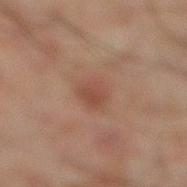follow-up: no biopsy performed (imaged during a skin exam) | image: 15 mm crop, total-body photography | site: the right lower leg | automated lesion analysis: a footprint of about 7 mm² and an outline eccentricity of about 0.45 (0 = round, 1 = elongated); a mean CIELAB color near L≈38 a*≈18 b*≈23 and a normalized lesion–skin contrast near 5 | diameter: ≈3.5 mm | illumination: cross-polarized | patient: male, aged 43–47.